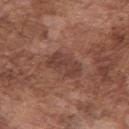biopsy status = catalogued during a skin exam; not biopsied
site = the right upper arm
lesion size = about 4 mm
tile lighting = white-light
image source = ~15 mm tile from a whole-body skin photo
patient = male, in their mid- to late 70s